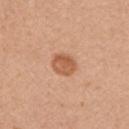Notes:
• location: the arm
• imaging modality: ~15 mm crop, total-body skin-cancer survey
• TBP lesion metrics: a lesion color around L≈57 a*≈24 b*≈35 in CIELAB, about 11 CIELAB-L* units darker than the surrounding skin, and a normalized lesion–skin contrast near 7.5
• subject: female, about 35 years old